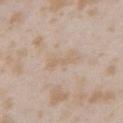Q: What are the patient's age and sex?
A: female, approximately 25 years of age
Q: How was this image acquired?
A: 15 mm crop, total-body photography
Q: What is the anatomic site?
A: the left upper arm
Q: What did automated image analysis measure?
A: a lesion color around L≈64 a*≈13 b*≈29 in CIELAB and a normalized border contrast of about 4.5; a nevus-likeness score of about 0/100
Q: How was the tile lit?
A: white-light